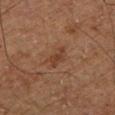Q: Is there a histopathology result?
A: catalogued during a skin exam; not biopsied
Q: What kind of image is this?
A: ~15 mm crop, total-body skin-cancer survey
Q: Lesion location?
A: the left lower leg
Q: What are the patient's age and sex?
A: male, roughly 75 years of age